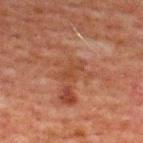Impression: Part of a total-body skin-imaging series; this lesion was reviewed on a skin check and was not flagged for biopsy. Background: An algorithmic analysis of the crop reported a border-irregularity index near 4/10. It also reported an automated nevus-likeness rating near 0 out of 100 and a detector confidence of about 100 out of 100 that the crop contains a lesion. From the upper back. A male subject about 60 years old. Cropped from a total-body skin-imaging series; the visible field is about 15 mm.